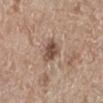Notes:
• notes · catalogued during a skin exam; not biopsied
• diameter · about 3 mm
• patient · female, aged approximately 70
• acquisition · ~15 mm crop, total-body skin-cancer survey
• location · the left lower leg
• TBP lesion metrics · a lesion area of about 5.5 mm² and an outline eccentricity of about 0.65 (0 = round, 1 = elongated); a lesion-to-skin contrast of about 9 (normalized; higher = more distinct)